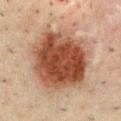Impression:
The lesion was tiled from a total-body skin photograph and was not biopsied.
Context:
Automated tile analysis of the lesion measured a lesion–skin lightness drop of about 14 and a normalized lesion–skin contrast near 12. The analysis additionally found a border-irregularity index near 1.5/10, a color-variation rating of about 6/10, and radial color variation of about 2. The software also gave an automated nevus-likeness rating near 100 out of 100. A roughly 15 mm field-of-view crop from a total-body skin photograph. On the chest. A male patient, aged around 50. The tile uses cross-polarized illumination.